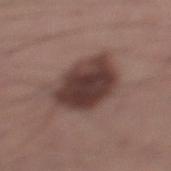Notes:
- notes · total-body-photography surveillance lesion; no biopsy
- lesion size · about 6 mm
- image source · 15 mm crop, total-body photography
- body site · the left lower leg
- tile lighting · white-light
- patient · male, aged 33 to 37